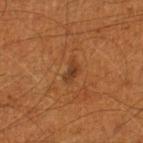From the left lower leg. A lesion tile, about 15 mm wide, cut from a 3D total-body photograph. A male subject, aged approximately 60. Imaged with cross-polarized lighting. About 3 mm across. The total-body-photography lesion software estimated a lesion area of about 4 mm², a shape eccentricity near 0.85, and a shape-asymmetry score of about 0.45 (0 = symmetric). The software also gave a mean CIELAB color near L≈32 a*≈19 b*≈30 and a lesion–skin lightness drop of about 6. The analysis additionally found an automated nevus-likeness rating near 0 out of 100 and lesion-presence confidence of about 100/100.